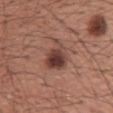{
  "biopsy_status": "not biopsied; imaged during a skin examination",
  "image": {
    "source": "total-body photography crop",
    "field_of_view_mm": 15
  },
  "patient": {
    "sex": "male",
    "age_approx": 45
  },
  "lesion_size": {
    "long_diameter_mm_approx": 4.0
  },
  "site": "mid back"
}